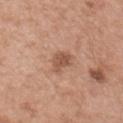lesion diameter: about 3.5 mm; image source: ~15 mm tile from a whole-body skin photo; lighting: white-light illumination; patient: male, roughly 55 years of age; anatomic site: the right upper arm.